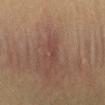notes=catalogued during a skin exam; not biopsied
location=the mid back
imaging modality=~15 mm tile from a whole-body skin photo
lesion diameter=≈2.5 mm
patient=female, aged around 50
image-analysis metrics=internal color variation of about 0 on a 0–10 scale and radial color variation of about 0; a nevus-likeness score of about 0/100
tile lighting=cross-polarized illumination